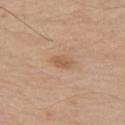Q: Was a biopsy performed?
A: catalogued during a skin exam; not biopsied
Q: What lighting was used for the tile?
A: white-light illumination
Q: Lesion size?
A: ≈2.5 mm
Q: How was this image acquired?
A: 15 mm crop, total-body photography
Q: Automated lesion metrics?
A: an area of roughly 3.5 mm², an eccentricity of roughly 0.8, and a symmetry-axis asymmetry near 0.2; a lesion color around L≈57 a*≈20 b*≈32 in CIELAB, a lesion–skin lightness drop of about 8, and a lesion-to-skin contrast of about 6 (normalized; higher = more distinct); border irregularity of about 2 on a 0–10 scale and peripheral color asymmetry of about 0.5
Q: What are the patient's age and sex?
A: male, in their mid- to late 50s
Q: Lesion location?
A: the upper back